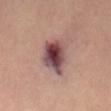Q: How was this image acquired?
A: total-body-photography crop, ~15 mm field of view
Q: How large is the lesion?
A: about 5 mm
Q: How was the tile lit?
A: cross-polarized illumination
Q: What are the patient's age and sex?
A: female, roughly 65 years of age
Q: Where on the body is the lesion?
A: the mid back